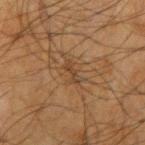Findings:
– follow-up — imaged on a skin check; not biopsied
– lesion diameter — about 2.5 mm
– subject — male, roughly 65 years of age
– location — the right upper arm
– image source — ~15 mm tile from a whole-body skin photo